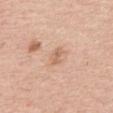Impression:
No biopsy was performed on this lesion — it was imaged during a full skin examination and was not determined to be concerning.
Acquisition and patient details:
This is a white-light tile. A lesion tile, about 15 mm wide, cut from a 3D total-body photograph. On the upper back. A male patient aged around 60.